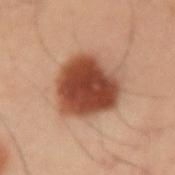  lesion_size:
    long_diameter_mm_approx: 6.0
  site: left upper arm
  image:
    source: total-body photography crop
    field_of_view_mm: 15
  patient:
    sex: male
    age_approx: 55
  lighting: cross-polarized